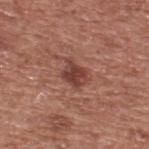Part of a total-body skin-imaging series; this lesion was reviewed on a skin check and was not flagged for biopsy. Imaged with white-light lighting. A 15 mm crop from a total-body photograph taken for skin-cancer surveillance. The lesion is located on the upper back. A male patient, in their mid-70s. The recorded lesion diameter is about 3 mm.